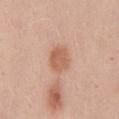Clinical impression: No biopsy was performed on this lesion — it was imaged during a full skin examination and was not determined to be concerning. Acquisition and patient details: The lesion is located on the back. The patient is a female about 40 years old. The recorded lesion diameter is about 3.5 mm. Automated image analysis of the tile measured a border-irregularity index near 1.5/10 and a peripheral color-asymmetry measure near 0.5. The software also gave a classifier nevus-likeness of about 85/100 and lesion-presence confidence of about 100/100. Captured under white-light illumination. A close-up tile cropped from a whole-body skin photograph, about 15 mm across.